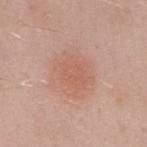follow-up: no biopsy performed (imaged during a skin exam); lesion size: ~5 mm (longest diameter); subject: male, aged around 55; image source: ~15 mm crop, total-body skin-cancer survey; tile lighting: white-light illumination; automated lesion analysis: a border-irregularity index near 3.5/10 and a peripheral color-asymmetry measure near 1; body site: the mid back.